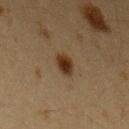{
  "biopsy_status": "not biopsied; imaged during a skin examination",
  "automated_metrics": {
    "cielab_L": 27,
    "cielab_a": 14,
    "cielab_b": 26,
    "vs_skin_darker_L": 10.0
  },
  "site": "left upper arm",
  "image": {
    "source": "total-body photography crop",
    "field_of_view_mm": 15
  },
  "lighting": "cross-polarized",
  "patient": {
    "sex": "female",
    "age_approx": 40
  },
  "lesion_size": {
    "long_diameter_mm_approx": 3.0
  }
}